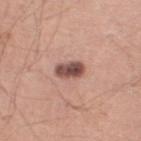The lesion was tiled from a total-body skin photograph and was not biopsied.
A roughly 15 mm field-of-view crop from a total-body skin photograph.
A male patient, approximately 40 years of age.
The lesion's longest dimension is about 4 mm.
Located on the left lower leg.